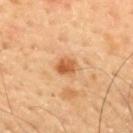Imaged during a routine full-body skin examination; the lesion was not biopsied and no histopathology is available.
The lesion is located on the mid back.
An algorithmic analysis of the crop reported border irregularity of about 1.5 on a 0–10 scale, internal color variation of about 4 on a 0–10 scale, and a peripheral color-asymmetry measure near 1.5. The software also gave a nevus-likeness score of about 80/100 and lesion-presence confidence of about 100/100.
Longest diameter approximately 3 mm.
A roughly 15 mm field-of-view crop from a total-body skin photograph.
The subject is a male aged 53–57.
The tile uses cross-polarized illumination.